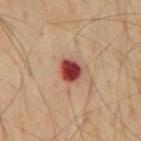Impression:
Recorded during total-body skin imaging; not selected for excision or biopsy.
Background:
A 15 mm close-up tile from a total-body photography series done for melanoma screening. The lesion's longest dimension is about 2.5 mm. A male patient, aged 53 to 57. The lesion is located on the back.